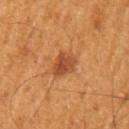No biopsy was performed on this lesion — it was imaged during a full skin examination and was not determined to be concerning.
A region of skin cropped from a whole-body photographic capture, roughly 15 mm wide.
Located on the left upper arm.
A male patient, about 60 years old.
Approximately 3.5 mm at its widest.
This is a cross-polarized tile.